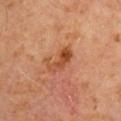Captured during whole-body skin photography for melanoma surveillance; the lesion was not biopsied. A male subject, aged approximately 60. Captured under cross-polarized illumination. Cropped from a total-body skin-imaging series; the visible field is about 15 mm. From the right upper arm.